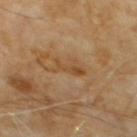The lesion was photographed on a routine skin check and not biopsied; there is no pathology result.
Automated tile analysis of the lesion measured a lesion area of about 5 mm² and two-axis asymmetry of about 0.4. The analysis additionally found an average lesion color of about L≈46 a*≈18 b*≈35 (CIELAB) and a normalized lesion–skin contrast near 5.5. It also reported border irregularity of about 5.5 on a 0–10 scale and a within-lesion color-variation index near 2.5/10.
Captured under cross-polarized illumination.
Cropped from a whole-body photographic skin survey; the tile spans about 15 mm.
From the chest.
The patient is a male approximately 60 years of age.